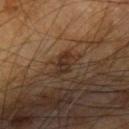Findings:
- biopsy status: catalogued during a skin exam; not biopsied
- automated lesion analysis: a lesion color around L≈31 a*≈17 b*≈26 in CIELAB and roughly 8 lightness units darker than nearby skin; a border-irregularity index near 2.5/10, a color-variation rating of about 3/10, and peripheral color asymmetry of about 1
- tile lighting: cross-polarized
- acquisition: total-body-photography crop, ~15 mm field of view
- subject: male, roughly 65 years of age
- anatomic site: the arm
- lesion diameter: about 3.5 mm